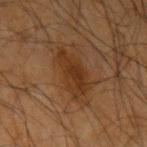Captured under cross-polarized illumination. This image is a 15 mm lesion crop taken from a total-body photograph. A male patient, aged approximately 65. The lesion is located on the left upper arm.